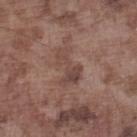Impression:
Part of a total-body skin-imaging series; this lesion was reviewed on a skin check and was not flagged for biopsy.
Clinical summary:
A male patient approximately 75 years of age. This is a white-light tile. The lesion's longest dimension is about 4.5 mm. Cropped from a whole-body photographic skin survey; the tile spans about 15 mm. From the left lower leg.